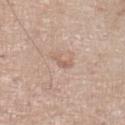Impression: Imaged during a routine full-body skin examination; the lesion was not biopsied and no histopathology is available.